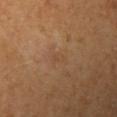Imaged during a routine full-body skin examination; the lesion was not biopsied and no histopathology is available.
The subject is a female roughly 55 years of age.
The lesion is on the left upper arm.
The recorded lesion diameter is about 2 mm.
Cropped from a whole-body photographic skin survey; the tile spans about 15 mm.
Captured under cross-polarized illumination.